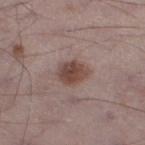Findings:
• notes: imaged on a skin check; not biopsied
• patient: male, aged approximately 70
• diameter: ~3.5 mm (longest diameter)
• image: 15 mm crop, total-body photography
• illumination: white-light illumination
• location: the left lower leg